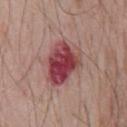Recorded during total-body skin imaging; not selected for excision or biopsy.
Automated image analysis of the tile measured a mean CIELAB color near L≈44 a*≈30 b*≈20, roughly 15 lightness units darker than nearby skin, and a lesion-to-skin contrast of about 11.5 (normalized; higher = more distinct). It also reported a classifier nevus-likeness of about 0/100.
The subject is a male aged approximately 70.
A 15 mm close-up tile from a total-body photography series done for melanoma screening.
Located on the chest.
The lesion's longest dimension is about 6 mm.